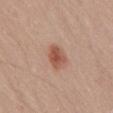| field | value |
|---|---|
| biopsy status | total-body-photography surveillance lesion; no biopsy |
| lighting | white-light illumination |
| image-analysis metrics | a lesion color around L≈53 a*≈24 b*≈30 in CIELAB and roughly 11 lightness units darker than nearby skin; a classifier nevus-likeness of about 100/100 and a lesion-detection confidence of about 100/100 |
| subject | male, aged around 65 |
| acquisition | total-body-photography crop, ~15 mm field of view |
| anatomic site | the lower back |
| lesion size | about 3 mm |